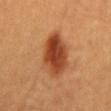notes: total-body-photography surveillance lesion; no biopsy
patient: female, in their 30s
lesion diameter: about 6 mm
image-analysis metrics: an outline eccentricity of about 0.8 (0 = round, 1 = elongated) and a symmetry-axis asymmetry near 0.15; border irregularity of about 2 on a 0–10 scale and a color-variation rating of about 6/10; a classifier nevus-likeness of about 100/100 and a lesion-detection confidence of about 100/100
acquisition: 15 mm crop, total-body photography
tile lighting: cross-polarized
location: the chest